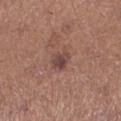Recorded during total-body skin imaging; not selected for excision or biopsy.
The subject is a female about 35 years old.
From the right lower leg.
Measured at roughly 2.5 mm in maximum diameter.
A 15 mm crop from a total-body photograph taken for skin-cancer surveillance.